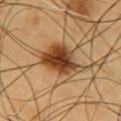Q: How was this image acquired?
A: total-body-photography crop, ~15 mm field of view
Q: How large is the lesion?
A: ~6 mm (longest diameter)
Q: What lighting was used for the tile?
A: cross-polarized
Q: Where on the body is the lesion?
A: the chest
Q: What are the patient's age and sex?
A: male, in their 60s
Q: Automated lesion metrics?
A: a footprint of about 13 mm², a shape eccentricity near 0.8, and a shape-asymmetry score of about 0.25 (0 = symmetric); roughly 17 lightness units darker than nearby skin and a normalized border contrast of about 13; a color-variation rating of about 7/10 and a peripheral color-asymmetry measure near 2; an automated nevus-likeness rating near 95 out of 100 and a lesion-detection confidence of about 100/100